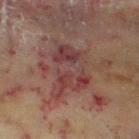biopsy status = imaged on a skin check; not biopsied | subject = female, approximately 60 years of age | lesion size = ≈6.5 mm | body site = the leg | imaging modality = ~15 mm tile from a whole-body skin photo | TBP lesion metrics = a lesion color around L≈36 a*≈21 b*≈19 in CIELAB, roughly 8 lightness units darker than nearby skin, and a normalized lesion–skin contrast near 7.5; a border-irregularity index near 5.5/10, a within-lesion color-variation index near 7.5/10, and peripheral color asymmetry of about 2.5; a classifier nevus-likeness of about 0/100 and a detector confidence of about 75 out of 100 that the crop contains a lesion | tile lighting = cross-polarized illumination.